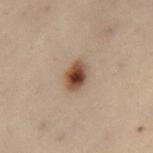Part of a total-body skin-imaging series; this lesion was reviewed on a skin check and was not flagged for biopsy. The lesion is located on the right thigh. The subject is a female aged 48 to 52. Measured at roughly 3.5 mm in maximum diameter. Cropped from a whole-body photographic skin survey; the tile spans about 15 mm.